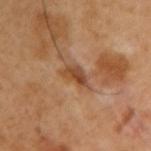This lesion was catalogued during total-body skin photography and was not selected for biopsy. A close-up tile cropped from a whole-body skin photograph, about 15 mm across. Longest diameter approximately 3.5 mm. From the right upper arm. Imaged with cross-polarized lighting. A male patient, aged around 50.